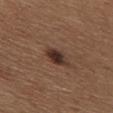Captured during whole-body skin photography for melanoma surveillance; the lesion was not biopsied. This is a white-light tile. The lesion-visualizer software estimated a lesion area of about 5 mm². The analysis additionally found a border-irregularity rating of about 1.5/10 and internal color variation of about 4.5 on a 0–10 scale. About 3 mm across. A 15 mm crop from a total-body photograph taken for skin-cancer surveillance. A male patient, in their mid- to late 60s. From the chest.